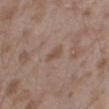This lesion was catalogued during total-body skin photography and was not selected for biopsy.
The lesion is on the right thigh.
A 15 mm close-up tile from a total-body photography series done for melanoma screening.
Automated tile analysis of the lesion measured a lesion area of about 3 mm², an outline eccentricity of about 0.85 (0 = round, 1 = elongated), and two-axis asymmetry of about 0.25. The software also gave a mean CIELAB color near L≈50 a*≈16 b*≈26, roughly 7 lightness units darker than nearby skin, and a normalized lesion–skin contrast near 5.5. The analysis additionally found border irregularity of about 2.5 on a 0–10 scale and radial color variation of about 0.
The tile uses white-light illumination.
The recorded lesion diameter is about 2.5 mm.
A male patient, approximately 45 years of age.